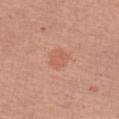The lesion was tiled from a total-body skin photograph and was not biopsied.
The subject is a female in their mid- to late 60s.
From the left forearm.
Cropped from a total-body skin-imaging series; the visible field is about 15 mm.